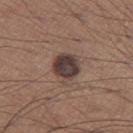Context: The total-body-photography lesion software estimated an area of roughly 8.5 mm² and two-axis asymmetry of about 0.25. It also reported a lesion color around L≈38 a*≈15 b*≈18 in CIELAB, roughly 14 lightness units darker than nearby skin, and a normalized lesion–skin contrast near 12. And it measured border irregularity of about 2 on a 0–10 scale, a within-lesion color-variation index near 4/10, and radial color variation of about 1. The analysis additionally found an automated nevus-likeness rating near 35 out of 100. A male subject, aged 63 to 67. Measured at roughly 3.5 mm in maximum diameter. This is a white-light tile. Located on the left thigh. A lesion tile, about 15 mm wide, cut from a 3D total-body photograph.